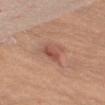Assessment: This lesion was catalogued during total-body skin photography and was not selected for biopsy. Background: Automated tile analysis of the lesion measured an area of roughly 5 mm² and an eccentricity of roughly 0.75. The analysis additionally found a classifier nevus-likeness of about 25/100 and a detector confidence of about 100 out of 100 that the crop contains a lesion. A region of skin cropped from a whole-body photographic capture, roughly 15 mm wide. On the right upper arm. A male patient, aged 73 to 77. The recorded lesion diameter is about 3 mm.